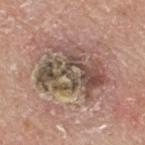Captured during whole-body skin photography for melanoma surveillance; the lesion was not biopsied. The lesion is on the upper back. A male patient approximately 80 years of age. Automated image analysis of the tile measured a border-irregularity index near 6.5/10 and a color-variation rating of about 10/10. The analysis additionally found a nevus-likeness score of about 0/100 and a detector confidence of about 70 out of 100 that the crop contains a lesion. Cropped from a whole-body photographic skin survey; the tile spans about 15 mm. Approximately 7.5 mm at its widest.